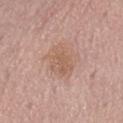Context: The tile uses white-light illumination. On the abdomen. A female patient about 50 years old. A close-up tile cropped from a whole-body skin photograph, about 15 mm across.